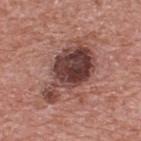{"biopsy_status": "not biopsied; imaged during a skin examination", "lesion_size": {"long_diameter_mm_approx": 7.5}, "lighting": "white-light", "image": {"source": "total-body photography crop", "field_of_view_mm": 15}, "automated_metrics": {"area_mm2_approx": 21.0, "eccentricity": 0.85, "shape_asymmetry": 0.4, "cielab_L": 40, "cielab_a": 22, "cielab_b": 22, "vs_skin_darker_L": 16.0, "vs_skin_contrast_norm": 12.5, "border_irregularity_0_10": 5.0, "color_variation_0_10": 6.5}, "patient": {"sex": "male", "age_approx": 70}, "site": "upper back"}